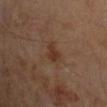This lesion was catalogued during total-body skin photography and was not selected for biopsy. A patient in their mid-50s. Located on the right upper arm. The tile uses cross-polarized illumination. A close-up tile cropped from a whole-body skin photograph, about 15 mm across. Approximately 3 mm at its widest.